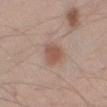illumination: white-light | patient: male, in their mid-40s | image source: ~15 mm tile from a whole-body skin photo | image-analysis metrics: an eccentricity of roughly 0.45 and two-axis asymmetry of about 0.25; border irregularity of about 2 on a 0–10 scale and a color-variation rating of about 2.5/10; an automated nevus-likeness rating near 95 out of 100 and a lesion-detection confidence of about 100/100 | lesion diameter: about 3 mm | site: the arm.